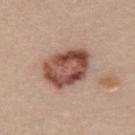follow-up: no biopsy performed (imaged during a skin exam); image source: 15 mm crop, total-body photography; lesion diameter: about 6 mm; tile lighting: white-light illumination; body site: the upper back; subject: female, aged 23 to 27.